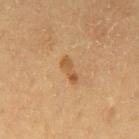Clinical impression:
Imaged during a routine full-body skin examination; the lesion was not biopsied and no histopathology is available.
Background:
The subject is a male in their 60s. This is a cross-polarized tile. From the mid back. Measured at roughly 3.5 mm in maximum diameter. A 15 mm close-up tile from a total-body photography series done for melanoma screening.